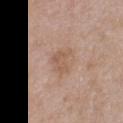The lesion was tiled from a total-body skin photograph and was not biopsied. A male subject approximately 50 years of age. A roughly 15 mm field-of-view crop from a total-body skin photograph. The lesion's longest dimension is about 3.5 mm. Imaged with white-light lighting. The lesion is on the chest.